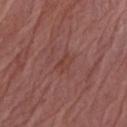No biopsy was performed on this lesion — it was imaged during a full skin examination and was not determined to be concerning. The subject is a female aged 63 to 67. This is a white-light tile. Located on the right forearm. Cropped from a total-body skin-imaging series; the visible field is about 15 mm. Approximately 3 mm at its widest.